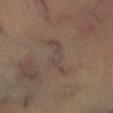Q: Was this lesion biopsied?
A: catalogued during a skin exam; not biopsied
Q: Patient demographics?
A: male, roughly 45 years of age
Q: How was the tile lit?
A: cross-polarized
Q: What kind of image is this?
A: 15 mm crop, total-body photography
Q: Lesion size?
A: ~5 mm (longest diameter)
Q: Where on the body is the lesion?
A: the right lower leg